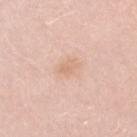No biopsy was performed on this lesion — it was imaged during a full skin examination and was not determined to be concerning.